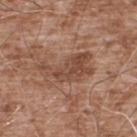Clinical impression:
Part of a total-body skin-imaging series; this lesion was reviewed on a skin check and was not flagged for biopsy.
Context:
A male subject, approximately 55 years of age. Imaged with white-light lighting. A close-up tile cropped from a whole-body skin photograph, about 15 mm across. The lesion's longest dimension is about 6.5 mm. An algorithmic analysis of the crop reported a lesion area of about 13 mm² and an eccentricity of roughly 0.85. The software also gave a color-variation rating of about 3/10 and a peripheral color-asymmetry measure near 1. The software also gave a classifier nevus-likeness of about 0/100. The lesion is on the upper back.